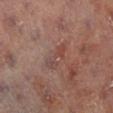Notes:
– notes — no biopsy performed (imaged during a skin exam)
– site — the left leg
– size — about 3.5 mm
– tile lighting — cross-polarized
– subject — female, in their 80s
– TBP lesion metrics — a mean CIELAB color near L≈40 a*≈19 b*≈21 and a lesion-to-skin contrast of about 5 (normalized; higher = more distinct); a classifier nevus-likeness of about 0/100 and a lesion-detection confidence of about 100/100
– image source — ~15 mm crop, total-body skin-cancer survey